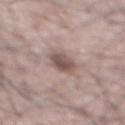Clinical impression: Imaged during a routine full-body skin examination; the lesion was not biopsied and no histopathology is available. Background: About 3 mm across. A region of skin cropped from a whole-body photographic capture, roughly 15 mm wide. A male subject, in their mid- to late 60s. The lesion is on the abdomen.